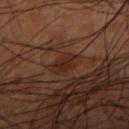Recorded during total-body skin imaging; not selected for excision or biopsy.
From the right upper arm.
Cropped from a whole-body photographic skin survey; the tile spans about 15 mm.
The subject is a male aged approximately 45.
Captured under cross-polarized illumination.
Automated tile analysis of the lesion measured about 6 CIELAB-L* units darker than the surrounding skin and a lesion-to-skin contrast of about 6.5 (normalized; higher = more distinct). The analysis additionally found a color-variation rating of about 1.5/10.